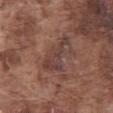Assessment: Imaged during a routine full-body skin examination; the lesion was not biopsied and no histopathology is available. Background: The lesion is located on the abdomen. A roughly 15 mm field-of-view crop from a total-body skin photograph. A male patient, aged 73–77. Captured under white-light illumination. About 5 mm across.